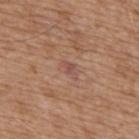workup = total-body-photography surveillance lesion; no biopsy
lighting = white-light
lesion diameter = about 2.5 mm
site = the upper back
image source = 15 mm crop, total-body photography
subject = male, aged around 75